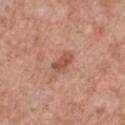workup=total-body-photography surveillance lesion; no biopsy | anatomic site=the front of the torso | image-analysis metrics=an area of roughly 4 mm² and two-axis asymmetry of about 0.25; a mean CIELAB color near L≈53 a*≈23 b*≈30, a lesion–skin lightness drop of about 10, and a normalized border contrast of about 7 | patient=male, aged 58 to 62 | illumination=white-light | acquisition=total-body-photography crop, ~15 mm field of view.